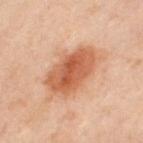The recorded lesion diameter is about 6.5 mm. Automated tile analysis of the lesion measured an outline eccentricity of about 0.8 (0 = round, 1 = elongated) and a symmetry-axis asymmetry near 0.15. The software also gave a mean CIELAB color near L≈57 a*≈25 b*≈35, about 13 CIELAB-L* units darker than the surrounding skin, and a lesion-to-skin contrast of about 9 (normalized; higher = more distinct). The analysis additionally found a within-lesion color-variation index near 5/10. The patient is a female aged 48–52. Located on the right upper arm. A 15 mm crop from a total-body photograph taken for skin-cancer surveillance. The tile uses cross-polarized illumination.